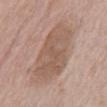* workup: imaged on a skin check; not biopsied
* acquisition: ~15 mm tile from a whole-body skin photo
* location: the abdomen
* subject: male, approximately 70 years of age